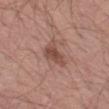This lesion was catalogued during total-body skin photography and was not selected for biopsy. On the left thigh. Automated tile analysis of the lesion measured a border-irregularity rating of about 3.5/10, a color-variation rating of about 2.5/10, and peripheral color asymmetry of about 1. The subject is a male approximately 70 years of age. The tile uses white-light illumination. A lesion tile, about 15 mm wide, cut from a 3D total-body photograph. About 4 mm across.